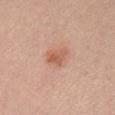This lesion was catalogued during total-body skin photography and was not selected for biopsy. The subject is a female aged around 25. Located on the chest. Cropped from a total-body skin-imaging series; the visible field is about 15 mm.